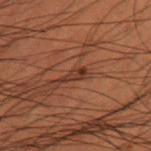The lesion was tiled from a total-body skin photograph and was not biopsied.
Cropped from a total-body skin-imaging series; the visible field is about 15 mm.
Captured under cross-polarized illumination.
About 2.5 mm across.
A male subject roughly 50 years of age.
On the leg.